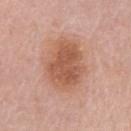Impression:
This lesion was catalogued during total-body skin photography and was not selected for biopsy.
Context:
Measured at roughly 6.5 mm in maximum diameter. A male patient, about 80 years old. The tile uses white-light illumination. A lesion tile, about 15 mm wide, cut from a 3D total-body photograph. The lesion is located on the chest.